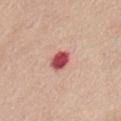notes: imaged on a skin check; not biopsied | location: the abdomen | diameter: ≈2.5 mm | subject: female, in their mid- to late 60s | acquisition: ~15 mm tile from a whole-body skin photo | tile lighting: white-light | image-analysis metrics: an area of roughly 5 mm² and a shape-asymmetry score of about 0.2 (0 = symmetric); a mean CIELAB color near L≈52 a*≈36 b*≈24, a lesion–skin lightness drop of about 19, and a normalized border contrast of about 12; a detector confidence of about 100 out of 100 that the crop contains a lesion.